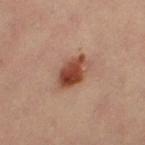Impression: The lesion was photographed on a routine skin check and not biopsied; there is no pathology result. Background: The lesion is located on the left thigh. This is a cross-polarized tile. The patient is a female aged approximately 35. A 15 mm crop from a total-body photograph taken for skin-cancer surveillance. Measured at roughly 4.5 mm in maximum diameter.